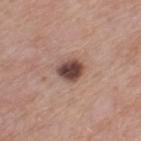Q: Was this lesion biopsied?
A: no biopsy performed (imaged during a skin exam)
Q: What is the anatomic site?
A: the left thigh
Q: What is the imaging modality?
A: ~15 mm tile from a whole-body skin photo
Q: What are the patient's age and sex?
A: male, about 75 years old
Q: What is the lesion's diameter?
A: about 3 mm
Q: Illumination type?
A: white-light illumination